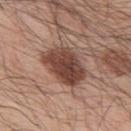workup — total-body-photography surveillance lesion; no biopsy
automated lesion analysis — a lesion area of about 15 mm² and a shape eccentricity near 0.75; a mean CIELAB color near L≈43 a*≈21 b*≈25, about 15 CIELAB-L* units darker than the surrounding skin, and a lesion-to-skin contrast of about 11.5 (normalized; higher = more distinct); border irregularity of about 2 on a 0–10 scale and internal color variation of about 4.5 on a 0–10 scale
subject — male, roughly 55 years of age
image source — ~15 mm tile from a whole-body skin photo
size — ≈5 mm
body site — the upper back
illumination — white-light